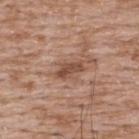Background: Imaged with white-light lighting. On the upper back. A male subject, aged approximately 50. Cropped from a total-body skin-imaging series; the visible field is about 15 mm.